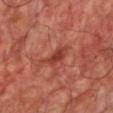No biopsy was performed on this lesion — it was imaged during a full skin examination and was not determined to be concerning.
The subject is a male in their mid-50s.
From the chest.
About 4.5 mm across.
This is a cross-polarized tile.
Automated tile analysis of the lesion measured an average lesion color of about L≈42 a*≈30 b*≈31 (CIELAB) and roughly 9 lightness units darker than nearby skin. The analysis additionally found a classifier nevus-likeness of about 0/100 and a detector confidence of about 100 out of 100 that the crop contains a lesion.
A lesion tile, about 15 mm wide, cut from a 3D total-body photograph.